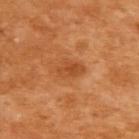Clinical impression:
The lesion was photographed on a routine skin check and not biopsied; there is no pathology result.
Acquisition and patient details:
From the back. A female subject about 55 years old. Longest diameter approximately 3.5 mm. This image is a 15 mm lesion crop taken from a total-body photograph. The lesion-visualizer software estimated a within-lesion color-variation index near 2.5/10 and peripheral color asymmetry of about 1.